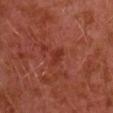Acquisition and patient details: An algorithmic analysis of the crop reported an area of roughly 3 mm², a shape eccentricity near 0.8, and a shape-asymmetry score of about 0.45 (0 = symmetric). The analysis additionally found roughly 6 lightness units darker than nearby skin. And it measured a border-irregularity rating of about 4/10, a within-lesion color-variation index near 0.5/10, and peripheral color asymmetry of about 0. The software also gave a nevus-likeness score of about 0/100 and a lesion-detection confidence of about 100/100. A 15 mm crop from a total-body photograph taken for skin-cancer surveillance. The tile uses cross-polarized illumination. The patient is a male approximately 30 years of age. The lesion is on the left arm.